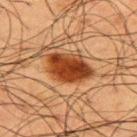<lesion>
<biopsy_status>not biopsied; imaged during a skin examination</biopsy_status>
<image>
  <source>total-body photography crop</source>
  <field_of_view_mm>15</field_of_view_mm>
</image>
<lighting>cross-polarized</lighting>
<patient>
  <sex>male</sex>
  <age_approx>60</age_approx>
</patient>
<site>upper back</site>
<lesion_size>
  <long_diameter_mm_approx>5.0</long_diameter_mm_approx>
</lesion_size>
<automated_metrics>
  <eccentricity>0.75</eccentricity>
  <border_irregularity_0_10>2.0</border_irregularity_0_10>
  <color_variation_0_10>4.5</color_variation_0_10>
  <peripheral_color_asymmetry>1.5</peripheral_color_asymmetry>
</automated_metrics>
</lesion>